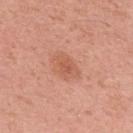No biopsy was performed on this lesion — it was imaged during a full skin examination and was not determined to be concerning.
A male subject roughly 55 years of age.
Approximately 3 mm at its widest.
A lesion tile, about 15 mm wide, cut from a 3D total-body photograph.
The total-body-photography lesion software estimated a lesion–skin lightness drop of about 8. The software also gave a lesion-detection confidence of about 100/100.
The lesion is located on the upper back.